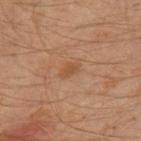<record>
<biopsy_status>not biopsied; imaged during a skin examination</biopsy_status>
<lesion_size>
  <long_diameter_mm_approx>2.5</long_diameter_mm_approx>
</lesion_size>
<patient>
  <sex>male</sex>
  <age_approx>30</age_approx>
</patient>
<site>left leg</site>
<lighting>cross-polarized</lighting>
<image>
  <source>total-body photography crop</source>
  <field_of_view_mm>15</field_of_view_mm>
</image>
</record>